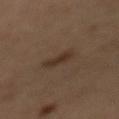Case summary:
- lesion size — ~3 mm (longest diameter)
- illumination — cross-polarized
- location — the mid back
- subject — male, roughly 60 years of age
- image — ~15 mm tile from a whole-body skin photo